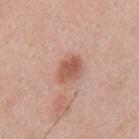follow-up = imaged on a skin check; not biopsied | subject = male, roughly 55 years of age | imaging modality = total-body-photography crop, ~15 mm field of view | site = the mid back.